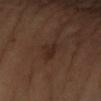Clinical impression:
Part of a total-body skin-imaging series; this lesion was reviewed on a skin check and was not flagged for biopsy.
Acquisition and patient details:
Captured under cross-polarized illumination. From the left forearm. A female subject, aged around 60. A roughly 15 mm field-of-view crop from a total-body skin photograph.